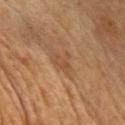Assessment:
The lesion was tiled from a total-body skin photograph and was not biopsied.
Image and clinical context:
The subject is a male about 65 years old. The tile uses cross-polarized illumination. A region of skin cropped from a whole-body photographic capture, roughly 15 mm wide. Approximately 4.5 mm at its widest. The lesion is located on the chest.